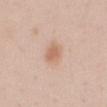– lesion diameter · about 2.5 mm
– tile lighting · white-light illumination
– body site · the abdomen
– patient · female, aged around 25
– image source · 15 mm crop, total-body photography
– TBP lesion metrics · a footprint of about 4.5 mm² and a shape-asymmetry score of about 0.2 (0 = symmetric); a border-irregularity index near 1.5/10 and internal color variation of about 1.5 on a 0–10 scale; a classifier nevus-likeness of about 95/100 and lesion-presence confidence of about 100/100